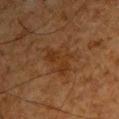<record>
<site>upper back</site>
<image>
  <source>total-body photography crop</source>
  <field_of_view_mm>15</field_of_view_mm>
</image>
<automated_metrics>
  <area_mm2_approx>11.0</area_mm2_approx>
  <eccentricity>0.7</eccentricity>
  <shape_asymmetry>0.45</shape_asymmetry>
  <border_irregularity_0_10>5.5</border_irregularity_0_10>
  <color_variation_0_10>2.5</color_variation_0_10>
  <peripheral_color_asymmetry>1.0</peripheral_color_asymmetry>
  <lesion_detection_confidence_0_100>100</lesion_detection_confidence_0_100>
</automated_metrics>
<lesion_size>
  <long_diameter_mm_approx>5.0</long_diameter_mm_approx>
</lesion_size>
<patient>
  <sex>male</sex>
  <age_approx>65</age_approx>
</patient>
</record>